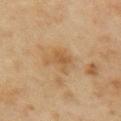{"biopsy_status": "not biopsied; imaged during a skin examination", "lesion_size": {"long_diameter_mm_approx": 4.0}, "site": "right upper arm", "lighting": "cross-polarized", "image": {"source": "total-body photography crop", "field_of_view_mm": 15}, "patient": {"sex": "female", "age_approx": 60}}